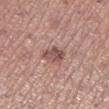Impression:
The lesion was photographed on a routine skin check and not biopsied; there is no pathology result.
Background:
From the left lower leg. The recorded lesion diameter is about 3 mm. The total-body-photography lesion software estimated a shape eccentricity near 0.65 and two-axis asymmetry of about 0.2. And it measured a mean CIELAB color near L≈51 a*≈21 b*≈22 and a lesion-to-skin contrast of about 8 (normalized; higher = more distinct). The analysis additionally found a border-irregularity index near 2/10, a within-lesion color-variation index near 4.5/10, and a peripheral color-asymmetry measure near 1.5. A female subject, in their mid- to late 60s. Captured under white-light illumination. A roughly 15 mm field-of-view crop from a total-body skin photograph.